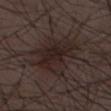Notes:
• diameter: ~7.5 mm (longest diameter)
• image: ~15 mm crop, total-body skin-cancer survey
• patient: male, aged approximately 50
• TBP lesion metrics: a border-irregularity rating of about 5/10 and a within-lesion color-variation index near 4/10
• lighting: white-light illumination